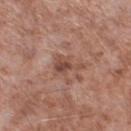The patient is a male in their mid- to late 50s.
A 15 mm close-up tile from a total-body photography series done for melanoma screening.
Located on the left lower leg.